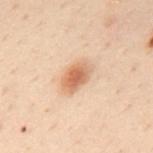No biopsy was performed on this lesion — it was imaged during a full skin examination and was not determined to be concerning. Longest diameter approximately 4 mm. A close-up tile cropped from a whole-body skin photograph, about 15 mm across. This is a cross-polarized tile. The patient is a male aged around 50. Located on the mid back.